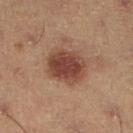follow-up=imaged on a skin check; not biopsied
location=the left lower leg
diameter=about 4.5 mm
image=~15 mm tile from a whole-body skin photo
illumination=cross-polarized illumination
TBP lesion metrics=a mean CIELAB color near L≈36 a*≈20 b*≈23, a lesion–skin lightness drop of about 11, and a normalized lesion–skin contrast near 9.5; a border-irregularity index near 1/10, internal color variation of about 3.5 on a 0–10 scale, and a peripheral color-asymmetry measure near 1; an automated nevus-likeness rating near 100 out of 100 and a detector confidence of about 100 out of 100 that the crop contains a lesion
subject=male, approximately 55 years of age